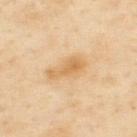follow-up — imaged on a skin check; not biopsied | imaging modality — total-body-photography crop, ~15 mm field of view | body site — the upper back | patient — male, roughly 55 years of age | TBP lesion metrics — a lesion color around L≈68 a*≈17 b*≈42 in CIELAB and a lesion–skin lightness drop of about 9; a classifier nevus-likeness of about 5/100 and lesion-presence confidence of about 100/100.